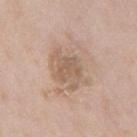Q: Was a biopsy performed?
A: imaged on a skin check; not biopsied
Q: Who is the patient?
A: male, approximately 65 years of age
Q: Where on the body is the lesion?
A: the mid back
Q: What lighting was used for the tile?
A: white-light
Q: What did automated image analysis measure?
A: a mean CIELAB color near L≈57 a*≈17 b*≈29, roughly 7 lightness units darker than nearby skin, and a normalized border contrast of about 5; a border-irregularity index near 6/10, internal color variation of about 2 on a 0–10 scale, and a peripheral color-asymmetry measure near 0.5; an automated nevus-likeness rating near 0 out of 100 and lesion-presence confidence of about 100/100
Q: How was this image acquired?
A: 15 mm crop, total-body photography